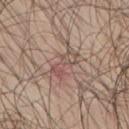* workup: catalogued during a skin exam; not biopsied
* automated lesion analysis: an outline eccentricity of about 0.9 (0 = round, 1 = elongated) and a symmetry-axis asymmetry near 0.3; an average lesion color of about L≈52 a*≈18 b*≈23 (CIELAB), a lesion–skin lightness drop of about 8, and a normalized border contrast of about 5.5
* diameter: ~3.5 mm (longest diameter)
* tile lighting: white-light illumination
* patient: male, about 70 years old
* acquisition: 15 mm crop, total-body photography
* site: the mid back